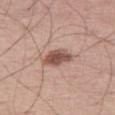This lesion was catalogued during total-body skin photography and was not selected for biopsy.
The lesion's longest dimension is about 4 mm.
This is a white-light tile.
A roughly 15 mm field-of-view crop from a total-body skin photograph.
An algorithmic analysis of the crop reported a border-irregularity index near 2.5/10, a color-variation rating of about 4/10, and peripheral color asymmetry of about 1.5.
Located on the leg.
A male patient, aged approximately 70.